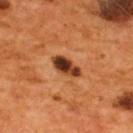Impression: The lesion was tiled from a total-body skin photograph and was not biopsied. Clinical summary: The lesion is located on the upper back. A 15 mm close-up tile from a total-body photography series done for melanoma screening. A male subject aged approximately 55. Imaged with cross-polarized lighting. Approximately 4 mm at its widest.